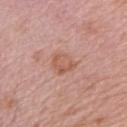Notes:
• workup — catalogued during a skin exam; not biopsied
• acquisition — ~15 mm crop, total-body skin-cancer survey
• anatomic site — the arm
• size — about 3 mm
• patient — female, in their mid-60s
• TBP lesion metrics — a within-lesion color-variation index near 3/10 and a peripheral color-asymmetry measure near 1; a classifier nevus-likeness of about 15/100